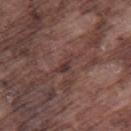follow-up=imaged on a skin check; not biopsied
lighting=white-light illumination
image-analysis metrics=a footprint of about 2.5 mm², a shape eccentricity near 0.85, and two-axis asymmetry of about 0.5; an average lesion color of about L≈35 a*≈19 b*≈19 (CIELAB), a lesion–skin lightness drop of about 7, and a normalized border contrast of about 7; a nevus-likeness score of about 0/100 and a detector confidence of about 70 out of 100 that the crop contains a lesion
site=the right thigh
image=~15 mm tile from a whole-body skin photo
patient=male, in their mid- to late 70s